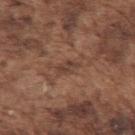Notes:
• follow-up · total-body-photography surveillance lesion; no biopsy